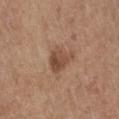Q: Was a biopsy performed?
A: catalogued during a skin exam; not biopsied
Q: How was this image acquired?
A: ~15 mm crop, total-body skin-cancer survey
Q: Who is the patient?
A: female, approximately 65 years of age
Q: What is the anatomic site?
A: the leg
Q: Automated lesion metrics?
A: a footprint of about 7 mm², a shape eccentricity near 0.75, and two-axis asymmetry of about 0.4; an average lesion color of about L≈48 a*≈19 b*≈29 (CIELAB) and a lesion-to-skin contrast of about 7.5 (normalized; higher = more distinct); a border-irregularity rating of about 4/10 and internal color variation of about 4 on a 0–10 scale